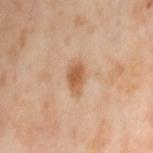Q: Was a biopsy performed?
A: catalogued during a skin exam; not biopsied
Q: Where on the body is the lesion?
A: the leg
Q: What is the imaging modality?
A: total-body-photography crop, ~15 mm field of view
Q: Who is the patient?
A: female, approximately 55 years of age